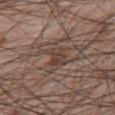Findings:
* notes: total-body-photography surveillance lesion; no biopsy
* anatomic site: the upper back
* tile lighting: white-light illumination
* image source: total-body-photography crop, ~15 mm field of view
* size: ~3 mm (longest diameter)
* patient: male, aged 63–67